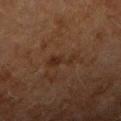Approximately 3.5 mm at its widest. A 15 mm crop from a total-body photograph taken for skin-cancer surveillance. The tile uses cross-polarized illumination. A female patient, aged 58–62. From the right upper arm.